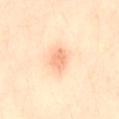No biopsy was performed on this lesion — it was imaged during a full skin examination and was not determined to be concerning.